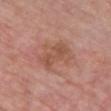  biopsy_status: not biopsied; imaged during a skin examination
  site: chest
  patient:
    sex: female
    age_approx: 65
  lighting: white-light
  automated_metrics:
    area_mm2_approx: 6.0
    eccentricity: 0.9
    shape_asymmetry: 0.3
    nevus_likeness_0_100: 0
    lesion_detection_confidence_0_100: 100
  image:
    source: total-body photography crop
    field_of_view_mm: 15
  lesion_size:
    long_diameter_mm_approx: 4.0